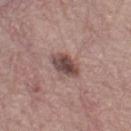Imaged during a routine full-body skin examination; the lesion was not biopsied and no histopathology is available. Automated tile analysis of the lesion measured a footprint of about 7 mm², an outline eccentricity of about 0.7 (0 = round, 1 = elongated), and a shape-asymmetry score of about 0.2 (0 = symmetric). The analysis additionally found a mean CIELAB color near L≈46 a*≈19 b*≈20, roughly 14 lightness units darker than nearby skin, and a normalized lesion–skin contrast near 10. It also reported a border-irregularity index near 1.5/10. Approximately 3.5 mm at its widest. A female patient in their mid-60s. Imaged with white-light lighting. A 15 mm crop from a total-body photograph taken for skin-cancer surveillance. The lesion is located on the left thigh.